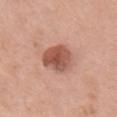{
  "biopsy_status": "not biopsied; imaged during a skin examination",
  "site": "chest",
  "patient": {
    "sex": "female",
    "age_approx": 65
  },
  "automated_metrics": {
    "area_mm2_approx": 12.0,
    "eccentricity": 0.65,
    "shape_asymmetry": 0.15,
    "cielab_L": 54,
    "cielab_a": 25,
    "cielab_b": 29,
    "vs_skin_darker_L": 14.0,
    "vs_skin_contrast_norm": 9.0,
    "border_irregularity_0_10": 1.5,
    "peripheral_color_asymmetry": 1.5,
    "nevus_likeness_0_100": 90
  },
  "image": {
    "source": "total-body photography crop",
    "field_of_view_mm": 15
  },
  "lighting": "white-light",
  "lesion_size": {
    "long_diameter_mm_approx": 4.5
  }
}